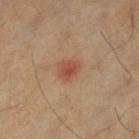Impression:
No biopsy was performed on this lesion — it was imaged during a full skin examination and was not determined to be concerning.
Clinical summary:
The patient is a male aged 68 to 72. A close-up tile cropped from a whole-body skin photograph, about 15 mm across. The lesion is located on the leg.